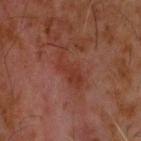No biopsy was performed on this lesion — it was imaged during a full skin examination and was not determined to be concerning. The total-body-photography lesion software estimated an eccentricity of roughly 0.95 and two-axis asymmetry of about 0.5. The software also gave a classifier nevus-likeness of about 0/100 and lesion-presence confidence of about 100/100. A lesion tile, about 15 mm wide, cut from a 3D total-body photograph. Longest diameter approximately 5.5 mm. The tile uses cross-polarized illumination. A male patient, aged approximately 60. From the head or neck.